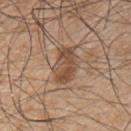This lesion was catalogued during total-body skin photography and was not selected for biopsy. A male subject in their 50s. Captured under white-light illumination. Measured at roughly 4.5 mm in maximum diameter. On the chest. A region of skin cropped from a whole-body photographic capture, roughly 15 mm wide.